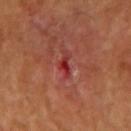{
  "image": {
    "source": "total-body photography crop",
    "field_of_view_mm": 15
  },
  "patient": {
    "sex": "female",
    "age_approx": 60
  },
  "site": "arm"
}